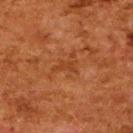Findings:
* biopsy status: no biopsy performed (imaged during a skin exam)
* lesion size: about 2.5 mm
* image source: 15 mm crop, total-body photography
* anatomic site: the upper back
* illumination: cross-polarized
* subject: female, in their 50s